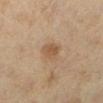Clinical impression: This lesion was catalogued during total-body skin photography and was not selected for biopsy. Image and clinical context: From the leg. A lesion tile, about 15 mm wide, cut from a 3D total-body photograph. Imaged with cross-polarized lighting. A female patient, about 40 years old. Automated tile analysis of the lesion measured a lesion area of about 5.5 mm², a shape eccentricity near 0.55, and a symmetry-axis asymmetry near 0.25. The analysis additionally found a classifier nevus-likeness of about 55/100 and a detector confidence of about 100 out of 100 that the crop contains a lesion.